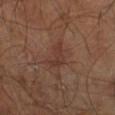Assessment:
No biopsy was performed on this lesion — it was imaged during a full skin examination and was not determined to be concerning.
Image and clinical context:
The lesion is on the leg. The lesion's longest dimension is about 3.5 mm. Imaged with cross-polarized lighting. Automated image analysis of the tile measured a border-irregularity rating of about 5.5/10, a color-variation rating of about 2/10, and radial color variation of about 0.5. The analysis additionally found a lesion-detection confidence of about 100/100. A lesion tile, about 15 mm wide, cut from a 3D total-body photograph. A male patient, approximately 60 years of age.